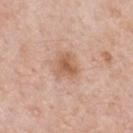Q: Was a biopsy performed?
A: total-body-photography surveillance lesion; no biopsy
Q: Where on the body is the lesion?
A: the mid back
Q: What did automated image analysis measure?
A: a mean CIELAB color near L≈59 a*≈21 b*≈32 and about 10 CIELAB-L* units darker than the surrounding skin; border irregularity of about 3 on a 0–10 scale, internal color variation of about 3.5 on a 0–10 scale, and peripheral color asymmetry of about 1; lesion-presence confidence of about 100/100
Q: Lesion size?
A: ~3 mm (longest diameter)
Q: What are the patient's age and sex?
A: male, aged 48–52
Q: What is the imaging modality?
A: total-body-photography crop, ~15 mm field of view